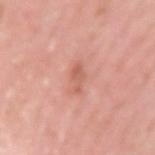Notes:
– image source: total-body-photography crop, ~15 mm field of view
– patient: female, aged 38 to 42
– location: the left upper arm